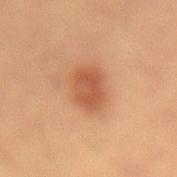Notes:
- notes — total-body-photography surveillance lesion; no biopsy
- automated metrics — an average lesion color of about L≈47 a*≈23 b*≈32 (CIELAB) and a lesion–skin lightness drop of about 9; a border-irregularity rating of about 1.5/10, a within-lesion color-variation index near 2.5/10, and radial color variation of about 1; a classifier nevus-likeness of about 100/100
- diameter — ~4 mm (longest diameter)
- subject — male, roughly 55 years of age
- tile lighting — cross-polarized
- site — the abdomen
- acquisition — 15 mm crop, total-body photography